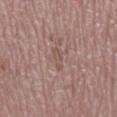Recorded during total-body skin imaging; not selected for excision or biopsy.
About 3 mm across.
Captured under white-light illumination.
A lesion tile, about 15 mm wide, cut from a 3D total-body photograph.
On the right lower leg.
The subject is a female about 65 years old.
An algorithmic analysis of the crop reported a lesion–skin lightness drop of about 6 and a normalized lesion–skin contrast near 5. The analysis additionally found an automated nevus-likeness rating near 0 out of 100 and a lesion-detection confidence of about 55/100.